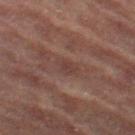The lesion was photographed on a routine skin check and not biopsied; there is no pathology result. The tile uses cross-polarized illumination. The lesion's longest dimension is about 2.5 mm. A female subject, approximately 70 years of age. Automated tile analysis of the lesion measured a lesion area of about 3 mm², an outline eccentricity of about 0.85 (0 = round, 1 = elongated), and a shape-asymmetry score of about 0.25 (0 = symmetric). And it measured an automated nevus-likeness rating near 0 out of 100 and a detector confidence of about 70 out of 100 that the crop contains a lesion. On the leg. A region of skin cropped from a whole-body photographic capture, roughly 15 mm wide.